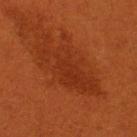Findings:
- workup · catalogued during a skin exam; not biopsied
- illumination · cross-polarized illumination
- lesion diameter · ~9 mm (longest diameter)
- subject · female, aged 28 to 32
- anatomic site · the right thigh
- imaging modality · 15 mm crop, total-body photography
- TBP lesion metrics · an eccentricity of roughly 0.95 and a shape-asymmetry score of about 0.45 (0 = symmetric); a mean CIELAB color near L≈31 a*≈30 b*≈36, roughly 6 lightness units darker than nearby skin, and a normalized lesion–skin contrast near 5.5; a border-irregularity rating of about 8/10 and a peripheral color-asymmetry measure near 0.5; a classifier nevus-likeness of about 0/100 and lesion-presence confidence of about 90/100